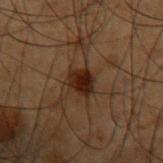The lesion was tiled from a total-body skin photograph and was not biopsied. Automated image analysis of the tile measured an area of roughly 5.5 mm². The software also gave a mean CIELAB color near L≈17 a*≈15 b*≈19, a lesion–skin lightness drop of about 9, and a lesion-to-skin contrast of about 12 (normalized; higher = more distinct). And it measured a within-lesion color-variation index near 3.5/10 and radial color variation of about 1. The software also gave a classifier nevus-likeness of about 90/100 and a detector confidence of about 100 out of 100 that the crop contains a lesion. The lesion's longest dimension is about 3 mm. A lesion tile, about 15 mm wide, cut from a 3D total-body photograph. A male subject about 50 years old. From the left forearm.